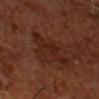Case summary:
- notes: no biopsy performed (imaged during a skin exam)
- TBP lesion metrics: a lesion area of about 11 mm² and a shape-asymmetry score of about 0.45 (0 = symmetric); an average lesion color of about L≈23 a*≈21 b*≈26 (CIELAB) and a normalized lesion–skin contrast near 6.5; a border-irregularity rating of about 8/10, a color-variation rating of about 2/10, and peripheral color asymmetry of about 0.5; a classifier nevus-likeness of about 0/100 and a lesion-detection confidence of about 100/100
- image: ~15 mm crop, total-body skin-cancer survey
- subject: male, aged around 65